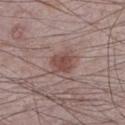| field | value |
|---|---|
| biopsy status | total-body-photography surveillance lesion; no biopsy |
| subject | male, in their mid-70s |
| site | the left lower leg |
| image source | ~15 mm tile from a whole-body skin photo |
| automated metrics | an average lesion color of about L≈48 a*≈20 b*≈21 (CIELAB), roughly 9 lightness units darker than nearby skin, and a lesion-to-skin contrast of about 7 (normalized; higher = more distinct); a classifier nevus-likeness of about 85/100 and lesion-presence confidence of about 100/100 |
| size | about 3.5 mm |